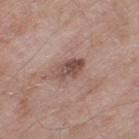Captured under white-light illumination.
Located on the right thigh.
A male subject aged 58 to 62.
The recorded lesion diameter is about 4 mm.
A 15 mm crop from a total-body photograph taken for skin-cancer surveillance.